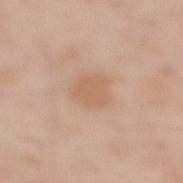Captured during whole-body skin photography for melanoma surveillance; the lesion was not biopsied.
A female patient, aged 48 to 52.
Located on the upper back.
A lesion tile, about 15 mm wide, cut from a 3D total-body photograph.
The recorded lesion diameter is about 3 mm.